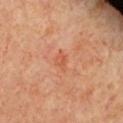Part of a total-body skin-imaging series; this lesion was reviewed on a skin check and was not flagged for biopsy.
A female patient aged 38–42.
The lesion is located on the chest.
A 15 mm crop from a total-body photograph taken for skin-cancer surveillance.